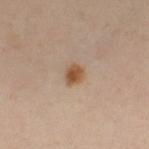Q: Was a biopsy performed?
A: catalogued during a skin exam; not biopsied
Q: What is the imaging modality?
A: total-body-photography crop, ~15 mm field of view
Q: How large is the lesion?
A: ≈2.5 mm
Q: Illumination type?
A: cross-polarized
Q: Patient demographics?
A: female, aged around 40
Q: Where on the body is the lesion?
A: the leg